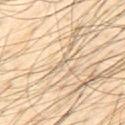| key | value |
|---|---|
| workup | no biopsy performed (imaged during a skin exam) |
| subject | male, approximately 40 years of age |
| automated lesion analysis | an area of roughly 1 mm², an outline eccentricity of about 0.9 (0 = round, 1 = elongated), and two-axis asymmetry of about 0.45; an average lesion color of about L≈70 a*≈11 b*≈32 (CIELAB), roughly 7 lightness units darker than nearby skin, and a lesion-to-skin contrast of about 4.5 (normalized; higher = more distinct) |
| location | the back |
| lighting | cross-polarized illumination |
| acquisition | 15 mm crop, total-body photography |
| size | ~1.5 mm (longest diameter) |